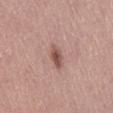The lesion was photographed on a routine skin check and not biopsied; there is no pathology result.